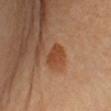Captured during whole-body skin photography for melanoma surveillance; the lesion was not biopsied. A female patient, aged 48 to 52. Captured under cross-polarized illumination. Approximately 3.5 mm at its widest. An algorithmic analysis of the crop reported an eccentricity of roughly 0.8 and a shape-asymmetry score of about 0.25 (0 = symmetric). The analysis additionally found a border-irregularity rating of about 3/10, a within-lesion color-variation index near 2/10, and radial color variation of about 0.5. The lesion is on the left upper arm. A 15 mm close-up extracted from a 3D total-body photography capture.